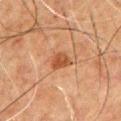Assessment: The lesion was photographed on a routine skin check and not biopsied; there is no pathology result. Image and clinical context: Measured at roughly 2.5 mm in maximum diameter. The subject is a male aged approximately 50. On the chest. Cropped from a whole-body photographic skin survey; the tile spans about 15 mm. The tile uses cross-polarized illumination.